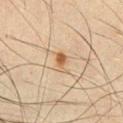The tile uses cross-polarized illumination.
A 15 mm close-up extracted from a 3D total-body photography capture.
A male patient, aged approximately 60.
Approximately 2.5 mm at its widest.
On the chest.
The total-body-photography lesion software estimated a lesion area of about 3 mm², a shape eccentricity near 0.85, and two-axis asymmetry of about 0.35. The software also gave a lesion color around L≈49 a*≈16 b*≈32 in CIELAB and a normalized lesion–skin contrast near 8.5. The analysis additionally found an automated nevus-likeness rating near 85 out of 100 and a lesion-detection confidence of about 100/100.